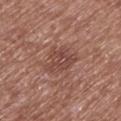workup: total-body-photography surveillance lesion; no biopsy
image source: total-body-photography crop, ~15 mm field of view
site: the leg
lighting: white-light
patient: male, aged approximately 75
image-analysis metrics: a within-lesion color-variation index near 3/10 and radial color variation of about 1